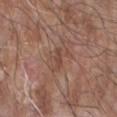Captured during whole-body skin photography for melanoma surveillance; the lesion was not biopsied.
Captured under white-light illumination.
Automated image analysis of the tile measured a lesion–skin lightness drop of about 7 and a normalized lesion–skin contrast near 5.5. The analysis additionally found border irregularity of about 4 on a 0–10 scale and radial color variation of about 0.
A close-up tile cropped from a whole-body skin photograph, about 15 mm across.
The lesion is on the right forearm.
Longest diameter approximately 2.5 mm.
The subject is a male aged approximately 60.